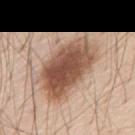• biopsy status: imaged on a skin check; not biopsied
• patient: male, aged approximately 80
• imaging modality: total-body-photography crop, ~15 mm field of view
• lighting: white-light
• site: the back
• lesion size: ~8 mm (longest diameter)